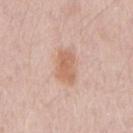Captured during whole-body skin photography for melanoma surveillance; the lesion was not biopsied.
A male subject aged 58 to 62.
From the arm.
This image is a 15 mm lesion crop taken from a total-body photograph.
Measured at roughly 3.5 mm in maximum diameter.
The lesion-visualizer software estimated a color-variation rating of about 2.5/10 and peripheral color asymmetry of about 1. It also reported a nevus-likeness score of about 55/100 and lesion-presence confidence of about 100/100.
This is a white-light tile.